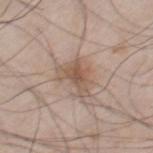Recorded during total-body skin imaging; not selected for excision or biopsy.
A lesion tile, about 15 mm wide, cut from a 3D total-body photograph.
Captured under white-light illumination.
Located on the right thigh.
A male subject in their 60s.